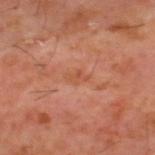Impression: Recorded during total-body skin imaging; not selected for excision or biopsy. Acquisition and patient details: Longest diameter approximately 2.5 mm. The patient is a male about 60 years old. The lesion is on the upper back. This is a cross-polarized tile. A lesion tile, about 15 mm wide, cut from a 3D total-body photograph.